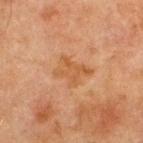Impression: Recorded during total-body skin imaging; not selected for excision or biopsy. Context: The lesion-visualizer software estimated a lesion color around L≈45 a*≈19 b*≈33 in CIELAB, about 6 CIELAB-L* units darker than the surrounding skin, and a normalized lesion–skin contrast near 6. Imaged with cross-polarized lighting. A male patient in their mid- to late 60s. This image is a 15 mm lesion crop taken from a total-body photograph. On the chest.